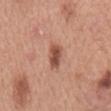{
  "biopsy_status": "not biopsied; imaged during a skin examination",
  "automated_metrics": {
    "area_mm2_approx": 5.5,
    "shape_asymmetry": 0.25,
    "vs_skin_darker_L": 13.0,
    "vs_skin_contrast_norm": 8.5
  },
  "site": "mid back",
  "lighting": "white-light",
  "image": {
    "source": "total-body photography crop",
    "field_of_view_mm": 15
  },
  "lesion_size": {
    "long_diameter_mm_approx": 3.5
  },
  "patient": {
    "sex": "male",
    "age_approx": 65
  }
}